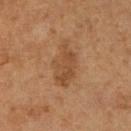notes: catalogued during a skin exam; not biopsied | patient: female, aged 63 to 67 | size: about 5.5 mm | imaging modality: ~15 mm crop, total-body skin-cancer survey | location: the right lower leg | illumination: cross-polarized.